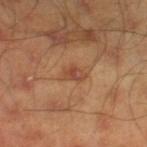notes: total-body-photography surveillance lesion; no biopsy | patient: male, aged 43 to 47 | image source: ~15 mm tile from a whole-body skin photo | automated lesion analysis: a lesion area of about 4 mm², a shape eccentricity near 0.75, and a symmetry-axis asymmetry near 0.25 | lesion size: about 2.5 mm | anatomic site: the right lower leg.